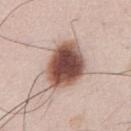Clinical impression:
No biopsy was performed on this lesion — it was imaged during a full skin examination and was not determined to be concerning.
Clinical summary:
Cropped from a total-body skin-imaging series; the visible field is about 15 mm. The lesion's longest dimension is about 6 mm. The lesion is located on the abdomen. The subject is a male in their mid- to late 30s.